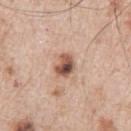<tbp_lesion>
<biopsy_status>not biopsied; imaged during a skin examination</biopsy_status>
<patient>
  <sex>male</sex>
  <age_approx>65</age_approx>
</patient>
<site>right upper arm</site>
<image>
  <source>total-body photography crop</source>
  <field_of_view_mm>15</field_of_view_mm>
</image>
<automated_metrics>
  <eccentricity>0.65</eccentricity>
  <shape_asymmetry>0.2</shape_asymmetry>
  <cielab_L>53</cielab_L>
  <cielab_a>20</cielab_a>
  <cielab_b>28</cielab_b>
  <vs_skin_darker_L>17.0</vs_skin_darker_L>
  <border_irregularity_0_10>2.0</border_irregularity_0_10>
  <peripheral_color_asymmetry>4.0</peripheral_color_asymmetry>
  <nevus_likeness_0_100>85</nevus_likeness_0_100>
  <lesion_detection_confidence_0_100>100</lesion_detection_confidence_0_100>
</automated_metrics>
<lesion_size>
  <long_diameter_mm_approx>3.0</long_diameter_mm_approx>
</lesion_size>
</tbp_lesion>